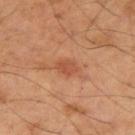- follow-up — catalogued during a skin exam; not biopsied
- imaging modality — 15 mm crop, total-body photography
- diameter — ~2.5 mm (longest diameter)
- location — the arm
- illumination — cross-polarized illumination
- subject — male, aged 48–52